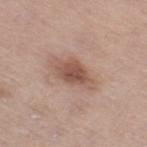Assessment:
This lesion was catalogued during total-body skin photography and was not selected for biopsy.
Acquisition and patient details:
Approximately 4.5 mm at its widest. The lesion is located on the right thigh. This is a white-light tile. A roughly 15 mm field-of-view crop from a total-body skin photograph. A female patient, aged 63 to 67.